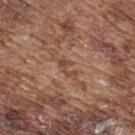A male patient, aged around 75. A 15 mm close-up tile from a total-body photography series done for melanoma screening. The lesion is on the upper back.